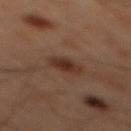{"biopsy_status": "not biopsied; imaged during a skin examination", "lighting": "cross-polarized", "image": {"source": "total-body photography crop", "field_of_view_mm": 15}, "site": "mid back", "lesion_size": {"long_diameter_mm_approx": 3.0}, "patient": {"sex": "male", "age_approx": 60}}